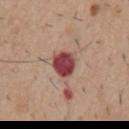location: the chest | image: total-body-photography crop, ~15 mm field of view | automated lesion analysis: an area of roughly 7 mm², an outline eccentricity of about 0.4 (0 = round, 1 = elongated), and a shape-asymmetry score of about 0.2 (0 = symmetric); border irregularity of about 1.5 on a 0–10 scale; a classifier nevus-likeness of about 0/100 and a lesion-detection confidence of about 100/100 | illumination: white-light illumination | patient: male, aged approximately 60 | diameter: ≈3 mm.